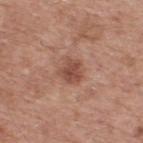Q: Was this lesion biopsied?
A: catalogued during a skin exam; not biopsied
Q: Automated lesion metrics?
A: an area of roughly 6 mm² and an eccentricity of roughly 0.25; border irregularity of about 2.5 on a 0–10 scale, a color-variation rating of about 3/10, and radial color variation of about 1; a classifier nevus-likeness of about 40/100 and a lesion-detection confidence of about 100/100
Q: How large is the lesion?
A: ≈3 mm
Q: Where on the body is the lesion?
A: the upper back
Q: How was this image acquired?
A: total-body-photography crop, ~15 mm field of view
Q: What are the patient's age and sex?
A: male, about 55 years old
Q: Illumination type?
A: white-light